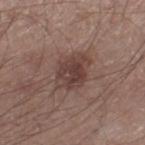follow-up = imaged on a skin check; not biopsied.